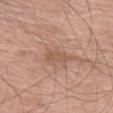follow-up: total-body-photography surveillance lesion; no biopsy.